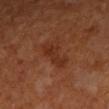This lesion was catalogued during total-body skin photography and was not selected for biopsy. Longest diameter approximately 5 mm. From the left forearm. A female patient roughly 65 years of age. A close-up tile cropped from a whole-body skin photograph, about 15 mm across.